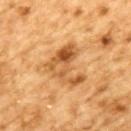workup: catalogued during a skin exam; not biopsied
lesion size: about 5 mm
site: the upper back
tile lighting: cross-polarized
TBP lesion metrics: a footprint of about 13 mm² and an outline eccentricity of about 0.65 (0 = round, 1 = elongated); a peripheral color-asymmetry measure near 3; a nevus-likeness score of about 45/100 and a detector confidence of about 100 out of 100 that the crop contains a lesion
image: 15 mm crop, total-body photography
subject: male, aged approximately 85 or older